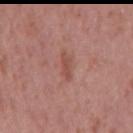Case summary:
* notes: imaged on a skin check; not biopsied
* illumination: white-light illumination
* site: the mid back
* image: ~15 mm tile from a whole-body skin photo
* subject: male, in their 70s
* diameter: ~2.5 mm (longest diameter)